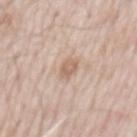{"biopsy_status": "not biopsied; imaged during a skin examination", "lighting": "white-light", "patient": {"sex": "male", "age_approx": 65}, "automated_metrics": {"area_mm2_approx": 3.5, "eccentricity": 0.75, "shape_asymmetry": 0.2, "border_irregularity_0_10": 2.0, "color_variation_0_10": 2.5, "peripheral_color_asymmetry": 1.0, "nevus_likeness_0_100": 0}, "image": {"source": "total-body photography crop", "field_of_view_mm": 15}, "site": "mid back"}